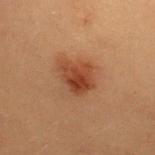  biopsy_status: not biopsied; imaged during a skin examination
  patient:
    sex: female
    age_approx: 20
  site: upper back
  lesion_size:
    long_diameter_mm_approx: 4.0
  image:
    source: total-body photography crop
    field_of_view_mm: 15